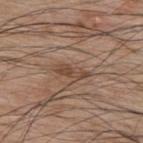No biopsy was performed on this lesion — it was imaged during a full skin examination and was not determined to be concerning.
This is a white-light tile.
Automated tile analysis of the lesion measured a footprint of about 4.5 mm² and an eccentricity of roughly 0.95. It also reported an average lesion color of about L≈46 a*≈17 b*≈27 (CIELAB), a lesion–skin lightness drop of about 9, and a lesion-to-skin contrast of about 7 (normalized; higher = more distinct). And it measured a border-irregularity rating of about 3.5/10, a within-lesion color-variation index near 4/10, and radial color variation of about 1.5.
A male subject about 70 years old.
Located on the upper back.
The recorded lesion diameter is about 4 mm.
A 15 mm close-up extracted from a 3D total-body photography capture.